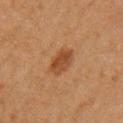This lesion was catalogued during total-body skin photography and was not selected for biopsy. This is a cross-polarized tile. A female patient, roughly 50 years of age. About 3.5 mm across. The lesion is located on the arm. A 15 mm crop from a total-body photograph taken for skin-cancer surveillance. Automated image analysis of the tile measured a lesion area of about 7 mm², a shape eccentricity near 0.75, and a symmetry-axis asymmetry near 0.15. It also reported roughly 8 lightness units darker than nearby skin and a lesion-to-skin contrast of about 7.5 (normalized; higher = more distinct).